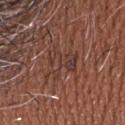No biopsy was performed on this lesion — it was imaged during a full skin examination and was not determined to be concerning. A male patient, roughly 45 years of age. Longest diameter approximately 4 mm. The lesion is located on the head or neck. A region of skin cropped from a whole-body photographic capture, roughly 15 mm wide. The lesion-visualizer software estimated border irregularity of about 7.5 on a 0–10 scale, internal color variation of about 7 on a 0–10 scale, and radial color variation of about 2.5. This is a white-light tile.